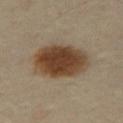Clinical impression: Recorded during total-body skin imaging; not selected for excision or biopsy. Background: The patient is a female aged approximately 40. On the right thigh. An algorithmic analysis of the crop reported about 15 CIELAB-L* units darker than the surrounding skin and a lesion-to-skin contrast of about 12 (normalized; higher = more distinct). And it measured border irregularity of about 1.5 on a 0–10 scale and a peripheral color-asymmetry measure near 1.5. The software also gave a classifier nevus-likeness of about 100/100 and a lesion-detection confidence of about 100/100. A 15 mm close-up extracted from a 3D total-body photography capture. Longest diameter approximately 7 mm.